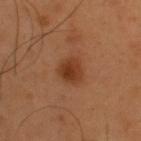image — ~15 mm crop, total-body skin-cancer survey; patient — male, approximately 55 years of age; location — the back.